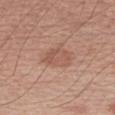Notes:
– notes — imaged on a skin check; not biopsied
– lesion diameter — ≈3.5 mm
– body site — the left forearm
– automated metrics — a lesion area of about 7 mm² and a shape eccentricity near 0.7; a lesion–skin lightness drop of about 7 and a normalized lesion–skin contrast near 5.5; an automated nevus-likeness rating near 20 out of 100 and a lesion-detection confidence of about 100/100
– lighting — white-light
– subject — male, aged approximately 60
– acquisition — 15 mm crop, total-body photography